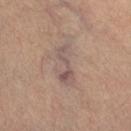biopsy status: total-body-photography surveillance lesion; no biopsy | tile lighting: cross-polarized | patient: female, aged around 40 | image source: ~15 mm crop, total-body skin-cancer survey | lesion diameter: ~5.5 mm (longest diameter) | image-analysis metrics: a border-irregularity rating of about 5/10, a within-lesion color-variation index near 5.5/10, and radial color variation of about 1.5 | anatomic site: the right lower leg.